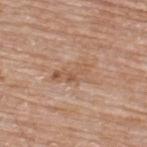Q: Was this lesion biopsied?
A: catalogued during a skin exam; not biopsied
Q: Who is the patient?
A: male, about 65 years old
Q: How was this image acquired?
A: ~15 mm crop, total-body skin-cancer survey
Q: Where on the body is the lesion?
A: the upper back